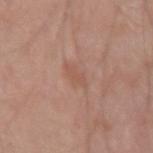Imaged during a routine full-body skin examination; the lesion was not biopsied and no histopathology is available. A male subject, in their mid-60s. Located on the right forearm. A lesion tile, about 15 mm wide, cut from a 3D total-body photograph.